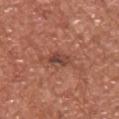{"biopsy_status": "not biopsied; imaged during a skin examination", "lesion_size": {"long_diameter_mm_approx": 3.0}, "image": {"source": "total-body photography crop", "field_of_view_mm": 15}, "site": "left upper arm", "patient": {"sex": "male", "age_approx": 45}, "lighting": "white-light"}